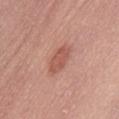The lesion was photographed on a routine skin check and not biopsied; there is no pathology result. The total-body-photography lesion software estimated an eccentricity of roughly 0.9 and two-axis asymmetry of about 0.2. The software also gave a lesion color around L≈55 a*≈25 b*≈28 in CIELAB, a lesion–skin lightness drop of about 9, and a normalized border contrast of about 6.5. It also reported a classifier nevus-likeness of about 70/100 and a lesion-detection confidence of about 100/100. A male patient, roughly 40 years of age. A roughly 15 mm field-of-view crop from a total-body skin photograph. Captured under white-light illumination. The lesion's longest dimension is about 4 mm. From the abdomen.